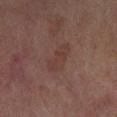| field | value |
|---|---|
| follow-up | no biopsy performed (imaged during a skin exam) |
| patient | female, roughly 60 years of age |
| imaging modality | total-body-photography crop, ~15 mm field of view |
| location | the left lower leg |